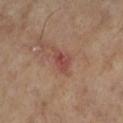Imaged during a routine full-body skin examination; the lesion was not biopsied and no histopathology is available. A region of skin cropped from a whole-body photographic capture, roughly 15 mm wide. The lesion's longest dimension is about 2.5 mm. Located on the right lower leg. This is a cross-polarized tile. A female patient roughly 75 years of age.